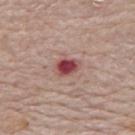This lesion was catalogued during total-body skin photography and was not selected for biopsy.
Captured under white-light illumination.
On the upper back.
A 15 mm close-up extracted from a 3D total-body photography capture.
A female patient aged 63–67.
The lesion's longest dimension is about 2.5 mm.